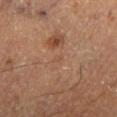Automated image analysis of the tile measured a lesion area of about 0.5 mm², an outline eccentricity of about 0.8 (0 = round, 1 = elongated), and two-axis asymmetry of about 0.35. The software also gave an automated nevus-likeness rating near 0 out of 100 and lesion-presence confidence of about 100/100. A male patient, about 60 years old. This image is a 15 mm lesion crop taken from a total-body photograph. Located on the left lower leg. About 1 mm across.